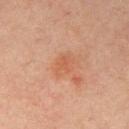This lesion was catalogued during total-body skin photography and was not selected for biopsy.
The lesion is on the right upper arm.
The recorded lesion diameter is about 3 mm.
An algorithmic analysis of the crop reported a nevus-likeness score of about 35/100 and a detector confidence of about 100 out of 100 that the crop contains a lesion.
A male subject aged 43–47.
A close-up tile cropped from a whole-body skin photograph, about 15 mm across.
This is a cross-polarized tile.